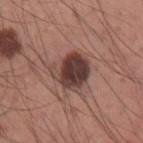Clinical impression:
No biopsy was performed on this lesion — it was imaged during a full skin examination and was not determined to be concerning.
Background:
A male patient aged 33–37. The tile uses white-light illumination. The lesion is located on the left upper arm. Automated tile analysis of the lesion measured an area of roughly 13 mm², an outline eccentricity of about 0.55 (0 = round, 1 = elongated), and a shape-asymmetry score of about 0.2 (0 = symmetric). It also reported a lesion color around L≈39 a*≈19 b*≈21 in CIELAB, a lesion–skin lightness drop of about 15, and a lesion-to-skin contrast of about 12 (normalized; higher = more distinct). The analysis additionally found border irregularity of about 3 on a 0–10 scale and internal color variation of about 5 on a 0–10 scale. And it measured a nevus-likeness score of about 20/100 and a detector confidence of about 100 out of 100 that the crop contains a lesion. This image is a 15 mm lesion crop taken from a total-body photograph. The recorded lesion diameter is about 4.5 mm.